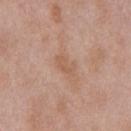  biopsy_status: not biopsied; imaged during a skin examination